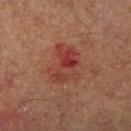Recorded during total-body skin imaging; not selected for excision or biopsy.
Longest diameter approximately 4.5 mm.
From the right lower leg.
The total-body-photography lesion software estimated a mean CIELAB color near L≈41 a*≈28 b*≈29, a lesion–skin lightness drop of about 8, and a normalized lesion–skin contrast near 6.5. The analysis additionally found a nevus-likeness score of about 0/100 and a detector confidence of about 100 out of 100 that the crop contains a lesion.
A male subject, approximately 65 years of age.
Cropped from a whole-body photographic skin survey; the tile spans about 15 mm.